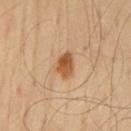A male patient, in their mid-40s. An algorithmic analysis of the crop reported an average lesion color of about L≈54 a*≈22 b*≈38 (CIELAB) and a lesion-to-skin contrast of about 9 (normalized; higher = more distinct). A lesion tile, about 15 mm wide, cut from a 3D total-body photograph. About 3.5 mm across. On the mid back. This is a cross-polarized tile.